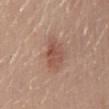  biopsy_status: not biopsied; imaged during a skin examination
  image:
    source: total-body photography crop
    field_of_view_mm: 15
  lesion_size:
    long_diameter_mm_approx: 4.0
  lighting: white-light
  site: lower back
  patient:
    sex: female
    age_approx: 40